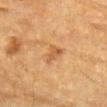Clinical impression: Imaged during a routine full-body skin examination; the lesion was not biopsied and no histopathology is available. Clinical summary: The lesion is on the left forearm. Imaged with cross-polarized lighting. A male subject, aged 83 or older. Longest diameter approximately 2.5 mm. A region of skin cropped from a whole-body photographic capture, roughly 15 mm wide.